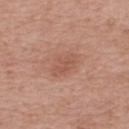Case summary:
– follow-up · total-body-photography surveillance lesion; no biopsy
– diameter · about 4 mm
– TBP lesion metrics · a footprint of about 7 mm², an outline eccentricity of about 0.75 (0 = round, 1 = elongated), and a symmetry-axis asymmetry near 0.2; an average lesion color of about L≈55 a*≈23 b*≈29 (CIELAB), about 7 CIELAB-L* units darker than the surrounding skin, and a normalized lesion–skin contrast near 5
– anatomic site · the back
– imaging modality · ~15 mm tile from a whole-body skin photo
– illumination · white-light illumination
– subject · male, in their mid-60s